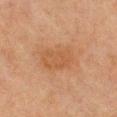biopsy status: imaged on a skin check; not biopsied
image: ~15 mm crop, total-body skin-cancer survey
illumination: cross-polarized
TBP lesion metrics: a lesion area of about 14 mm², an eccentricity of roughly 0.75, and a shape-asymmetry score of about 0.2 (0 = symmetric)
anatomic site: the chest
subject: female, aged 58 to 62
lesion diameter: ~5 mm (longest diameter)